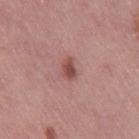Impression: This lesion was catalogued during total-body skin photography and was not selected for biopsy. Clinical summary: The lesion is located on the leg. A lesion tile, about 15 mm wide, cut from a 3D total-body photograph. The patient is a female about 50 years old.